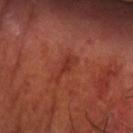follow-up: imaged on a skin check; not biopsied | subject: male, aged 68–72 | image: 15 mm crop, total-body photography | lighting: cross-polarized | body site: the arm | lesion size: about 3.5 mm.